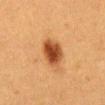follow-up=catalogued during a skin exam; not biopsied | site=the chest | lighting=cross-polarized | automated lesion analysis=a lesion area of about 10 mm² and an eccentricity of roughly 0.65; border irregularity of about 1.5 on a 0–10 scale and radial color variation of about 1.5 | patient=female, about 40 years old | image=total-body-photography crop, ~15 mm field of view.